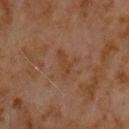follow-up = total-body-photography surveillance lesion; no biopsy | site = the chest | acquisition = total-body-photography crop, ~15 mm field of view | patient = male, aged around 60.